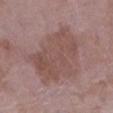Q: Was this lesion biopsied?
A: no biopsy performed (imaged during a skin exam)
Q: What is the anatomic site?
A: the left lower leg
Q: Illumination type?
A: white-light
Q: Who is the patient?
A: female, about 70 years old
Q: Lesion size?
A: ≈8 mm
Q: What is the imaging modality?
A: ~15 mm tile from a whole-body skin photo